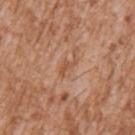The lesion was photographed on a routine skin check and not biopsied; there is no pathology result. The lesion is located on the left upper arm. The recorded lesion diameter is about 3 mm. The patient is a male roughly 45 years of age. A lesion tile, about 15 mm wide, cut from a 3D total-body photograph. Automated tile analysis of the lesion measured a footprint of about 3 mm² and a shape-asymmetry score of about 0.45 (0 = symmetric). And it measured border irregularity of about 6 on a 0–10 scale and radial color variation of about 0. It also reported a nevus-likeness score of about 0/100 and a lesion-detection confidence of about 100/100. Imaged with white-light lighting.